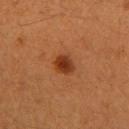Impression: Recorded during total-body skin imaging; not selected for excision or biopsy. Clinical summary: From the arm. The subject is a female in their 40s. The lesion-visualizer software estimated a mean CIELAB color near L≈32 a*≈25 b*≈33, about 11 CIELAB-L* units darker than the surrounding skin, and a normalized border contrast of about 9.5. The software also gave a detector confidence of about 100 out of 100 that the crop contains a lesion. A 15 mm crop from a total-body photograph taken for skin-cancer surveillance. This is a cross-polarized tile.